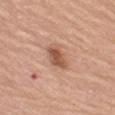The lesion was tiled from a total-body skin photograph and was not biopsied. The subject is a female aged 68–72. Located on the left thigh. Captured under white-light illumination. Longest diameter approximately 3.5 mm. The total-body-photography lesion software estimated an area of roughly 6 mm² and an eccentricity of roughly 0.85. The software also gave a mean CIELAB color near L≈55 a*≈22 b*≈31, about 12 CIELAB-L* units darker than the surrounding skin, and a lesion-to-skin contrast of about 8 (normalized; higher = more distinct). The analysis additionally found an automated nevus-likeness rating near 25 out of 100. A 15 mm close-up tile from a total-body photography series done for melanoma screening.